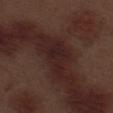| key | value |
|---|---|
| notes | total-body-photography surveillance lesion; no biopsy |